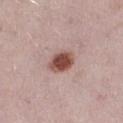A 15 mm close-up tile from a total-body photography series done for melanoma screening.
The patient is a female approximately 30 years of age.
From the right thigh.
Imaged with white-light lighting.